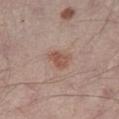Case summary:
• follow-up: total-body-photography surveillance lesion; no biopsy
• patient: male, roughly 55 years of age
• imaging modality: ~15 mm tile from a whole-body skin photo
• size: ≈3 mm
• location: the left thigh
• illumination: white-light